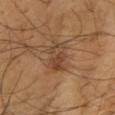Context:
The lesion's longest dimension is about 5.5 mm. Imaged with cross-polarized lighting. The patient is a male about 65 years old. A region of skin cropped from a whole-body photographic capture, roughly 15 mm wide. From the right upper arm. Automated tile analysis of the lesion measured a mean CIELAB color near L≈45 a*≈19 b*≈33, about 8 CIELAB-L* units darker than the surrounding skin, and a normalized lesion–skin contrast near 6.